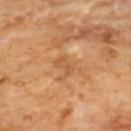Case summary:
– biopsy status · imaged on a skin check; not biopsied
– automated metrics · an area of roughly 3.5 mm² and an eccentricity of roughly 0.8; an average lesion color of about L≈57 a*≈24 b*≈41 (CIELAB), about 7 CIELAB-L* units darker than the surrounding skin, and a normalized lesion–skin contrast near 5; a border-irregularity index near 3/10, internal color variation of about 2.5 on a 0–10 scale, and radial color variation of about 0.5
– lighting · cross-polarized
– site · the upper back
– imaging modality · ~15 mm tile from a whole-body skin photo
– lesion size · ~2.5 mm (longest diameter)